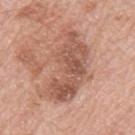No biopsy was performed on this lesion — it was imaged during a full skin examination and was not determined to be concerning. Located on the arm. The tile uses white-light illumination. Measured at roughly 9 mm in maximum diameter. A region of skin cropped from a whole-body photographic capture, roughly 15 mm wide. A male subject aged approximately 80. Automated image analysis of the tile measured an average lesion color of about L≈56 a*≈22 b*≈29 (CIELAB) and a normalized border contrast of about 7.